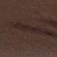Captured during whole-body skin photography for melanoma surveillance; the lesion was not biopsied.
On the left forearm.
This image is a 15 mm lesion crop taken from a total-body photograph.
A male patient, aged approximately 70.